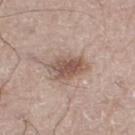Clinical impression:
The lesion was photographed on a routine skin check and not biopsied; there is no pathology result.
Context:
The lesion is located on the right leg. The patient is a male approximately 70 years of age. The lesion-visualizer software estimated a border-irregularity rating of about 2.5/10 and peripheral color asymmetry of about 1.5. The analysis additionally found a nevus-likeness score of about 80/100 and a detector confidence of about 100 out of 100 that the crop contains a lesion. Measured at roughly 4 mm in maximum diameter. This image is a 15 mm lesion crop taken from a total-body photograph.